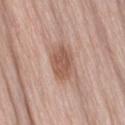This lesion was catalogued during total-body skin photography and was not selected for biopsy.
A female patient, roughly 60 years of age.
Automated image analysis of the tile measured two-axis asymmetry of about 0.2. It also reported internal color variation of about 2.5 on a 0–10 scale and radial color variation of about 1. It also reported a nevus-likeness score of about 70/100.
A 15 mm crop from a total-body photograph taken for skin-cancer surveillance.
This is a white-light tile.
Approximately 4 mm at its widest.
The lesion is located on the right thigh.